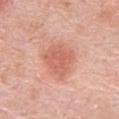Captured during whole-body skin photography for melanoma surveillance; the lesion was not biopsied. Cropped from a whole-body photographic skin survey; the tile spans about 15 mm. Automated image analysis of the tile measured a lesion area of about 13 mm², an outline eccentricity of about 0.4 (0 = round, 1 = elongated), and two-axis asymmetry of about 0.25. Measured at roughly 4.5 mm in maximum diameter. This is a white-light tile. A male patient, in their 80s. From the left upper arm.